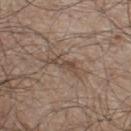<tbp_lesion>
  <biopsy_status>not biopsied; imaged during a skin examination</biopsy_status>
  <site>leg</site>
  <lighting>white-light</lighting>
  <automated_metrics>
    <cielab_L>47</cielab_L>
    <cielab_a>15</cielab_a>
    <cielab_b>24</cielab_b>
    <vs_skin_darker_L>8.0</vs_skin_darker_L>
    <vs_skin_contrast_norm>6.0</vs_skin_contrast_norm>
    <border_irregularity_0_10>6.5</border_irregularity_0_10>
    <peripheral_color_asymmetry>1.0</peripheral_color_asymmetry>
  </automated_metrics>
  <image>
    <source>total-body photography crop</source>
    <field_of_view_mm>15</field_of_view_mm>
  </image>
  <lesion_size>
    <long_diameter_mm_approx>4.5</long_diameter_mm_approx>
  </lesion_size>
  <patient>
    <sex>male</sex>
    <age_approx>75</age_approx>
  </patient>
</tbp_lesion>